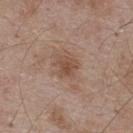Imaged during a routine full-body skin examination; the lesion was not biopsied and no histopathology is available. An algorithmic analysis of the crop reported a lesion area of about 5.5 mm², a shape eccentricity near 0.45, and a shape-asymmetry score of about 0.25 (0 = symmetric). It also reported a border-irregularity rating of about 3/10, a within-lesion color-variation index near 2.5/10, and peripheral color asymmetry of about 1. It also reported an automated nevus-likeness rating near 5 out of 100 and lesion-presence confidence of about 100/100. A region of skin cropped from a whole-body photographic capture, roughly 15 mm wide. The lesion is located on the upper back. A male subject, aged 53–57. Imaged with white-light lighting.